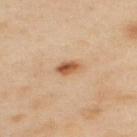{
  "biopsy_status": "not biopsied; imaged during a skin examination",
  "site": "upper back",
  "patient": {
    "sex": "male",
    "age_approx": 55
  },
  "image": {
    "source": "total-body photography crop",
    "field_of_view_mm": 15
  },
  "automated_metrics": {
    "area_mm2_approx": 3.5,
    "eccentricity": 0.75,
    "shape_asymmetry": 0.2,
    "border_irregularity_0_10": 2.0,
    "peripheral_color_asymmetry": 2.0,
    "nevus_likeness_0_100": 100,
    "lesion_detection_confidence_0_100": 100
  }
}